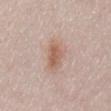No biopsy was performed on this lesion — it was imaged during a full skin examination and was not determined to be concerning. Imaged with white-light lighting. The lesion is on the abdomen. Cropped from a total-body skin-imaging series; the visible field is about 15 mm. Measured at roughly 4 mm in maximum diameter. The subject is a female aged around 50.